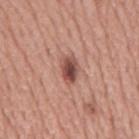The lesion was photographed on a routine skin check and not biopsied; there is no pathology result.
The tile uses white-light illumination.
Longest diameter approximately 3.5 mm.
A male subject about 75 years old.
On the mid back.
A roughly 15 mm field-of-view crop from a total-body skin photograph.